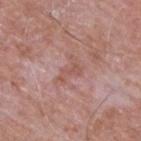biopsy_status: not biopsied; imaged during a skin examination
site: upper back
image:
  source: total-body photography crop
  field_of_view_mm: 15
lesion_size:
  long_diameter_mm_approx: 3.0
lighting: white-light
patient:
  sex: male
  age_approx: 65